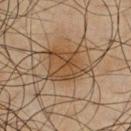Case summary:
- notes: imaged on a skin check; not biopsied
- subject: male, aged approximately 45
- illumination: cross-polarized illumination
- automated metrics: an average lesion color of about L≈38 a*≈14 b*≈28 (CIELAB) and a normalized lesion–skin contrast near 7.5; a classifier nevus-likeness of about 65/100 and a detector confidence of about 100 out of 100 that the crop contains a lesion
- location: the chest
- lesion size: ~6 mm (longest diameter)
- image: ~15 mm crop, total-body skin-cancer survey